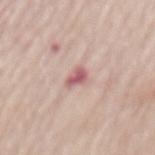Assessment:
Imaged during a routine full-body skin examination; the lesion was not biopsied and no histopathology is available.
Background:
A female subject, aged 63–67. The lesion-visualizer software estimated an average lesion color of about L≈59 a*≈26 b*≈20 (CIELAB), roughly 13 lightness units darker than nearby skin, and a normalized lesion–skin contrast near 8.5. Imaged with white-light lighting. Measured at roughly 2.5 mm in maximum diameter. The lesion is on the mid back. A lesion tile, about 15 mm wide, cut from a 3D total-body photograph.